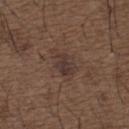The lesion's longest dimension is about 4.5 mm.
A roughly 15 mm field-of-view crop from a total-body skin photograph.
From the upper back.
The lesion-visualizer software estimated an eccentricity of roughly 0.85. And it measured an average lesion color of about L≈35 a*≈15 b*≈20 (CIELAB), about 7 CIELAB-L* units darker than the surrounding skin, and a normalized border contrast of about 7. It also reported a detector confidence of about 100 out of 100 that the crop contains a lesion.
A male subject approximately 50 years of age.